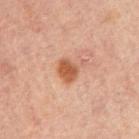Recorded during total-body skin imaging; not selected for excision or biopsy.
A lesion tile, about 15 mm wide, cut from a 3D total-body photograph.
The lesion is located on the right upper arm.
A male subject, roughly 30 years of age.
The recorded lesion diameter is about 3.5 mm.
Imaged with cross-polarized lighting.
An algorithmic analysis of the crop reported a lesion color around L≈46 a*≈21 b*≈30 in CIELAB and a lesion-to-skin contrast of about 8.5 (normalized; higher = more distinct). The analysis additionally found a classifier nevus-likeness of about 90/100 and lesion-presence confidence of about 100/100.